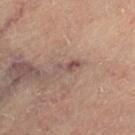{
  "biopsy_status": "not biopsied; imaged during a skin examination",
  "patient": {
    "sex": "female",
    "age_approx": 65
  },
  "lesion_size": {
    "long_diameter_mm_approx": 3.5
  },
  "lighting": "cross-polarized",
  "automated_metrics": {
    "area_mm2_approx": 3.5,
    "eccentricity": 0.95,
    "shape_asymmetry": 0.4,
    "cielab_L": 50,
    "cielab_a": 18,
    "cielab_b": 22,
    "vs_skin_darker_L": 9.0
  },
  "image": {
    "source": "total-body photography crop",
    "field_of_view_mm": 15
  },
  "site": "left thigh"
}